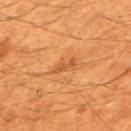Case summary:
– workup: imaged on a skin check; not biopsied
– subject: male, in their 60s
– automated lesion analysis: an area of roughly 2.5 mm², an outline eccentricity of about 0.95 (0 = round, 1 = elongated), and two-axis asymmetry of about 0.3
– imaging modality: ~15 mm crop, total-body skin-cancer survey
– location: the mid back